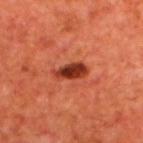| field | value |
|---|---|
| biopsy status | imaged on a skin check; not biopsied |
| acquisition | 15 mm crop, total-body photography |
| lesion size | ~4 mm (longest diameter) |
| illumination | cross-polarized |
| body site | the back |
| subject | male, approximately 70 years of age |